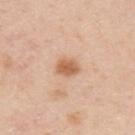This image is a 15 mm lesion crop taken from a total-body photograph.
Approximately 3 mm at its widest.
A male patient in their mid- to late 40s.
An algorithmic analysis of the crop reported a mean CIELAB color near L≈62 a*≈22 b*≈35, a lesion–skin lightness drop of about 12, and a normalized lesion–skin contrast near 8.
The lesion is located on the upper back.